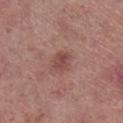image:
  source: total-body photography crop
  field_of_view_mm: 15
lesion_size:
  long_diameter_mm_approx: 2.5
patient:
  sex: male
  age_approx: 55
site: right lower leg
lighting: white-light
automated_metrics:
  area_mm2_approx: 4.5
  shape_asymmetry: 0.15
  border_irregularity_0_10: 1.5
  peripheral_color_asymmetry: 1.0
  nevus_likeness_0_100: 20
  lesion_detection_confidence_0_100: 100